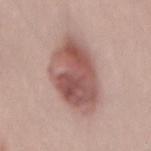Findings:
* acquisition · ~15 mm crop, total-body skin-cancer survey
* subject · male, roughly 25 years of age
* location · the lower back
* lesion diameter · ~7 mm (longest diameter)
* lighting · white-light